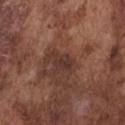Assessment: Imaged during a routine full-body skin examination; the lesion was not biopsied and no histopathology is available. Image and clinical context: A close-up tile cropped from a whole-body skin photograph, about 15 mm across. Approximately 3 mm at its widest. An algorithmic analysis of the crop reported a mean CIELAB color near L≈34 a*≈19 b*≈22, about 7 CIELAB-L* units darker than the surrounding skin, and a normalized border contrast of about 7.5. A male subject, aged around 75. On the chest. This is a white-light tile.